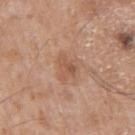Q: Is there a histopathology result?
A: no biopsy performed (imaged during a skin exam)
Q: How large is the lesion?
A: ≈3 mm
Q: Who is the patient?
A: male, in their 60s
Q: What did automated image analysis measure?
A: a normalized border contrast of about 5.5; border irregularity of about 3.5 on a 0–10 scale and a within-lesion color-variation index near 4/10; a nevus-likeness score of about 0/100 and lesion-presence confidence of about 100/100
Q: Illumination type?
A: white-light illumination
Q: What is the imaging modality?
A: ~15 mm crop, total-body skin-cancer survey
Q: What is the anatomic site?
A: the right upper arm